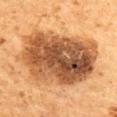{"lesion_size": {"long_diameter_mm_approx": 10.5}, "lighting": "cross-polarized", "image": {"source": "total-body photography crop", "field_of_view_mm": 15}, "patient": {"sex": "male", "age_approx": 60}, "site": "mid back", "automated_metrics": {"area_mm2_approx": 55.0, "eccentricity": 0.8, "shape_asymmetry": 0.15, "vs_skin_darker_L": 16.0, "vs_skin_contrast_norm": 12.0, "nevus_likeness_0_100": 5, "lesion_detection_confidence_0_100": 100}}